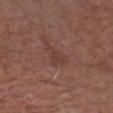Q: Is there a histopathology result?
A: catalogued during a skin exam; not biopsied